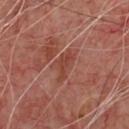This lesion was catalogued during total-body skin photography and was not selected for biopsy.
A 15 mm close-up extracted from a 3D total-body photography capture.
A male subject, aged 63 to 67.
Located on the chest.
The tile uses cross-polarized illumination.